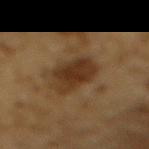Impression: Recorded during total-body skin imaging; not selected for excision or biopsy. Context: A region of skin cropped from a whole-body photographic capture, roughly 15 mm wide. Approximately 6 mm at its widest. A male patient, in their mid-80s or older. Captured under cross-polarized illumination. The lesion is on the back.